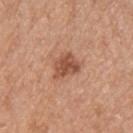The lesion is located on the upper back.
The subject is a male approximately 55 years of age.
Cropped from a total-body skin-imaging series; the visible field is about 15 mm.